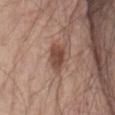Context: A male subject, aged around 80. A roughly 15 mm field-of-view crop from a total-body skin photograph. Captured under white-light illumination. Located on the abdomen. Approximately 4 mm at its widest.